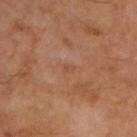Impression: Imaged during a routine full-body skin examination; the lesion was not biopsied and no histopathology is available. Background: The recorded lesion diameter is about 1.5 mm. Located on the upper back. Cropped from a whole-body photographic skin survey; the tile spans about 15 mm. The subject is a male in their mid- to late 50s. This is a cross-polarized tile.